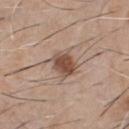| field | value |
|---|---|
| site | the chest |
| patient | male, about 60 years old |
| size | about 3.5 mm |
| image source | ~15 mm crop, total-body skin-cancer survey |
| lighting | white-light illumination |